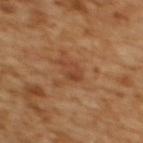The lesion was photographed on a routine skin check and not biopsied; there is no pathology result.
A region of skin cropped from a whole-body photographic capture, roughly 15 mm wide.
Located on the back.
A female subject aged approximately 55.
The recorded lesion diameter is about 2.5 mm.